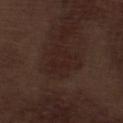Image and clinical context: The patient is a male aged 68 to 72. Located on the left lower leg. Cropped from a whole-body photographic skin survey; the tile spans about 15 mm. Captured under white-light illumination.